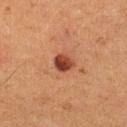Part of a total-body skin-imaging series; this lesion was reviewed on a skin check and was not flagged for biopsy.